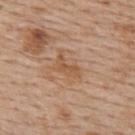Notes:
* workup: catalogued during a skin exam; not biopsied
* subject: female, aged around 65
* body site: the upper back
* automated lesion analysis: a footprint of about 4 mm² and a shape eccentricity near 0.85; a classifier nevus-likeness of about 0/100 and a detector confidence of about 100 out of 100 that the crop contains a lesion
* diameter: ~3 mm (longest diameter)
* imaging modality: ~15 mm tile from a whole-body skin photo